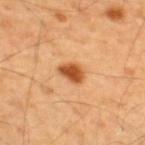Q: Is there a histopathology result?
A: total-body-photography surveillance lesion; no biopsy
Q: How was this image acquired?
A: total-body-photography crop, ~15 mm field of view
Q: What lighting was used for the tile?
A: cross-polarized
Q: How large is the lesion?
A: ≈3 mm
Q: Patient demographics?
A: male, aged 53–57
Q: What did automated image analysis measure?
A: a border-irregularity index near 2/10 and radial color variation of about 1; an automated nevus-likeness rating near 100 out of 100
Q: Lesion location?
A: the back